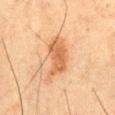workup=total-body-photography surveillance lesion; no biopsy
anatomic site=the chest
subject=male, aged 48–52
image=total-body-photography crop, ~15 mm field of view
lesion size=≈6 mm